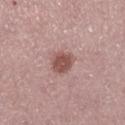The lesion was tiled from a total-body skin photograph and was not biopsied.
The recorded lesion diameter is about 3 mm.
Imaged with white-light lighting.
On the right lower leg.
Automated tile analysis of the lesion measured a footprint of about 6 mm² and an eccentricity of roughly 0.55. It also reported a lesion color around L≈51 a*≈22 b*≈23 in CIELAB, a lesion–skin lightness drop of about 12, and a lesion-to-skin contrast of about 8.5 (normalized; higher = more distinct). The analysis additionally found a within-lesion color-variation index near 2/10 and a peripheral color-asymmetry measure near 0.5.
The subject is a female aged approximately 50.
A lesion tile, about 15 mm wide, cut from a 3D total-body photograph.